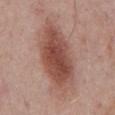Imaged during a routine full-body skin examination; the lesion was not biopsied and no histopathology is available. Automated image analysis of the tile measured a border-irregularity rating of about 2.5/10 and peripheral color asymmetry of about 1.5. The analysis additionally found a nevus-likeness score of about 95/100 and a detector confidence of about 100 out of 100 that the crop contains a lesion. Captured under white-light illumination. Cropped from a total-body skin-imaging series; the visible field is about 15 mm. The recorded lesion diameter is about 9.5 mm. A male patient in their 70s. On the chest.